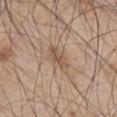Recorded during total-body skin imaging; not selected for excision or biopsy. A 15 mm close-up extracted from a 3D total-body photography capture. A male subject, aged 43 to 47. The lesion is on the right upper arm. The recorded lesion diameter is about 4 mm. The lesion-visualizer software estimated a lesion area of about 7 mm², an outline eccentricity of about 0.85 (0 = round, 1 = elongated), and a shape-asymmetry score of about 0.2 (0 = symmetric). And it measured an automated nevus-likeness rating near 0 out of 100 and lesion-presence confidence of about 100/100. The tile uses white-light illumination.